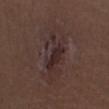The lesion was tiled from a total-body skin photograph and was not biopsied.
Cropped from a whole-body photographic skin survey; the tile spans about 15 mm.
This is a white-light tile.
Located on the left lower leg.
A male subject, aged 48–52.
The lesion's longest dimension is about 7.5 mm.
Automated image analysis of the tile measured a border-irregularity index near 4/10 and a peripheral color-asymmetry measure near 1.5. The analysis additionally found a classifier nevus-likeness of about 25/100 and a detector confidence of about 100 out of 100 that the crop contains a lesion.